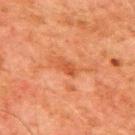site = the mid back; imaging modality = ~15 mm tile from a whole-body skin photo; lighting = cross-polarized; size = ≈2.5 mm; patient = male, aged approximately 80.